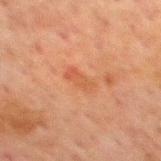Clinical impression:
The lesion was tiled from a total-body skin photograph and was not biopsied.
Clinical summary:
A close-up tile cropped from a whole-body skin photograph, about 15 mm across. The tile uses cross-polarized illumination. A male subject aged 58–62. The lesion is located on the mid back. About 3 mm across.